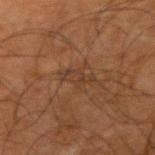The lesion was photographed on a routine skin check and not biopsied; there is no pathology result. The total-body-photography lesion software estimated a footprint of about 4.5 mm² and a shape eccentricity near 0.9. It also reported a mean CIELAB color near L≈30 a*≈16 b*≈25 and roughly 5 lightness units darker than nearby skin. A close-up tile cropped from a whole-body skin photograph, about 15 mm across. Located on the right upper arm. Approximately 3.5 mm at its widest. A male patient in their mid-60s. This is a cross-polarized tile.